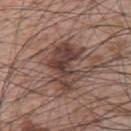Notes:
– anatomic site — the upper back
– subject — male, in their mid-60s
– lesion size — ~6 mm (longest diameter)
– imaging modality — ~15 mm tile from a whole-body skin photo